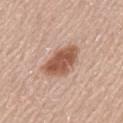Captured during whole-body skin photography for melanoma surveillance; the lesion was not biopsied. This is a white-light tile. A close-up tile cropped from a whole-body skin photograph, about 15 mm across. A male patient, approximately 60 years of age. The recorded lesion diameter is about 5 mm. Located on the mid back.